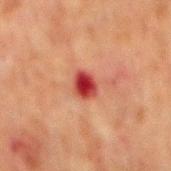Notes:
- follow-up — catalogued during a skin exam; not biopsied
- lighting — cross-polarized illumination
- diameter — ≈3 mm
- subject — male, aged approximately 60
- TBP lesion metrics — an eccentricity of roughly 0.65 and a shape-asymmetry score of about 0.2 (0 = symmetric); a border-irregularity rating of about 2/10; a nevus-likeness score of about 0/100 and a lesion-detection confidence of about 100/100
- acquisition — ~15 mm crop, total-body skin-cancer survey
- anatomic site — the back